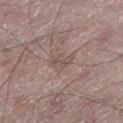biopsy status = no biopsy performed (imaged during a skin exam); illumination = white-light illumination; lesion size = ~3 mm (longest diameter); image = ~15 mm crop, total-body skin-cancer survey; patient = male, approximately 65 years of age; location = the left lower leg.